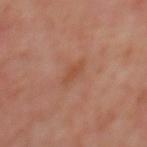<record>
  <patient>
    <sex>female</sex>
    <age_approx>50</age_approx>
  </patient>
  <image>
    <source>total-body photography crop</source>
    <field_of_view_mm>15</field_of_view_mm>
  </image>
  <site>upper back</site>
</record>